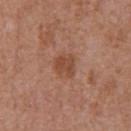Impression: The lesion was tiled from a total-body skin photograph and was not biopsied. Image and clinical context: The lesion is on the chest. Cropped from a whole-body photographic skin survey; the tile spans about 15 mm. A male subject, roughly 75 years of age. The lesion-visualizer software estimated a lesion area of about 6 mm² and a shape-asymmetry score of about 0.25 (0 = symmetric). And it measured an average lesion color of about L≈47 a*≈23 b*≈31 (CIELAB), roughly 8 lightness units darker than nearby skin, and a normalized lesion–skin contrast near 6.5. It also reported an automated nevus-likeness rating near 15 out of 100 and a lesion-detection confidence of about 100/100. Measured at roughly 3 mm in maximum diameter. The tile uses white-light illumination.